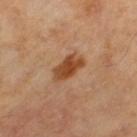This is a cross-polarized tile. An algorithmic analysis of the crop reported a lesion color around L≈45 a*≈23 b*≈35 in CIELAB and a lesion-to-skin contrast of about 9.5 (normalized; higher = more distinct). The analysis additionally found border irregularity of about 2.5 on a 0–10 scale and peripheral color asymmetry of about 1. It also reported a classifier nevus-likeness of about 90/100 and lesion-presence confidence of about 100/100. A male patient in their mid- to late 60s. A 15 mm close-up tile from a total-body photography series done for melanoma screening. Longest diameter approximately 4 mm.